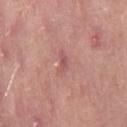Clinical impression: Recorded during total-body skin imaging; not selected for excision or biopsy. Context: The subject is a female aged approximately 50. Located on the leg. A lesion tile, about 15 mm wide, cut from a 3D total-body photograph.